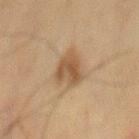Impression: Recorded during total-body skin imaging; not selected for excision or biopsy. Image and clinical context: Captured under cross-polarized illumination. Approximately 4 mm at its widest. A 15 mm crop from a total-body photograph taken for skin-cancer surveillance. The lesion is located on the mid back. A male subject aged around 70.